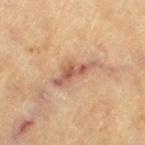follow-up — imaged on a skin check; not biopsied | patient — female, aged around 80 | site — the leg | acquisition — 15 mm crop, total-body photography | illumination — cross-polarized.